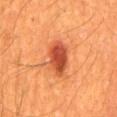follow-up — total-body-photography surveillance lesion; no biopsy
size — about 5 mm
image — 15 mm crop, total-body photography
patient — male, aged around 60
image-analysis metrics — a border-irregularity index near 2.5/10, a color-variation rating of about 5/10, and a peripheral color-asymmetry measure near 1.5; a classifier nevus-likeness of about 100/100
site — the mid back
lighting — cross-polarized illumination